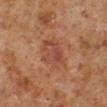  biopsy_status: not biopsied; imaged during a skin examination
  image:
    source: total-body photography crop
    field_of_view_mm: 15
  lesion_size:
    long_diameter_mm_approx: 3.5
  automated_metrics:
    area_mm2_approx: 10.0
    eccentricity: 0.55
    shape_asymmetry: 0.25
    cielab_L: 43
    cielab_a: 24
    cielab_b: 28
    vs_skin_darker_L: 7.0
    border_irregularity_0_10: 3.0
    color_variation_0_10: 4.5
    peripheral_color_asymmetry: 1.5
  patient:
    sex: male
    age_approx: 60
  lighting: cross-polarized
  site: left lower leg